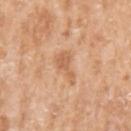The lesion was tiled from a total-body skin photograph and was not biopsied.
Longest diameter approximately 3.5 mm.
A female subject, approximately 75 years of age.
From the left upper arm.
This image is a 15 mm lesion crop taken from a total-body photograph.
This is a white-light tile.
Automated image analysis of the tile measured an area of roughly 5 mm², an eccentricity of roughly 0.85, and a symmetry-axis asymmetry near 0.65. And it measured a mean CIELAB color near L≈62 a*≈22 b*≈37 and a lesion–skin lightness drop of about 9. The analysis additionally found a border-irregularity rating of about 7/10 and a within-lesion color-variation index near 1.5/10. And it measured a nevus-likeness score of about 0/100 and a lesion-detection confidence of about 100/100.